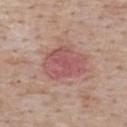Recorded during total-body skin imaging; not selected for excision or biopsy. The total-body-photography lesion software estimated a footprint of about 15 mm², a shape eccentricity near 0.7, and a symmetry-axis asymmetry near 0.2. It also reported a mean CIELAB color near L≈54 a*≈25 b*≈22, a lesion–skin lightness drop of about 9, and a normalized border contrast of about 6.5. Captured under white-light illumination. A male patient, in their mid- to late 70s. Located on the upper back. Longest diameter approximately 5 mm. A region of skin cropped from a whole-body photographic capture, roughly 15 mm wide.